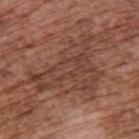Q: What did automated image analysis measure?
A: a lesion color around L≈41 a*≈21 b*≈27 in CIELAB, roughly 7 lightness units darker than nearby skin, and a lesion-to-skin contrast of about 6 (normalized; higher = more distinct); a border-irregularity rating of about 10/10 and a peripheral color-asymmetry measure near 1.5
Q: How large is the lesion?
A: ≈8.5 mm
Q: How was the tile lit?
A: white-light illumination
Q: What are the patient's age and sex?
A: male, aged 73 to 77
Q: Lesion location?
A: the upper back
Q: What kind of image is this?
A: ~15 mm tile from a whole-body skin photo